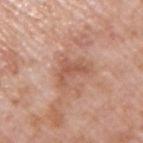Imaged during a routine full-body skin examination; the lesion was not biopsied and no histopathology is available.
Approximately 3.5 mm at its widest.
The lesion is located on the right upper arm.
A male subject aged 68–72.
Automated tile analysis of the lesion measured roughly 8 lightness units darker than nearby skin and a normalized lesion–skin contrast near 6. The analysis additionally found a border-irregularity rating of about 7/10 and peripheral color asymmetry of about 0.5.
A close-up tile cropped from a whole-body skin photograph, about 15 mm across.
This is a white-light tile.